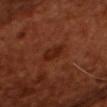Clinical impression: The lesion was tiled from a total-body skin photograph and was not biopsied. Clinical summary: The lesion's longest dimension is about 3.5 mm. A male patient, roughly 50 years of age. The lesion is on the head or neck. A region of skin cropped from a whole-body photographic capture, roughly 15 mm wide. Captured under cross-polarized illumination.